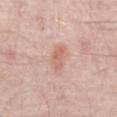Part of a total-body skin-imaging series; this lesion was reviewed on a skin check and was not flagged for biopsy. Measured at roughly 3 mm in maximum diameter. From the abdomen. A 15 mm close-up tile from a total-body photography series done for melanoma screening. The subject is in their mid-50s.